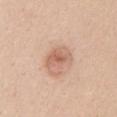biopsy status: total-body-photography surveillance lesion; no biopsy | body site: the mid back | automated lesion analysis: a footprint of about 7.5 mm² and an eccentricity of roughly 0.75 | illumination: white-light | acquisition: ~15 mm crop, total-body skin-cancer survey | subject: female, aged approximately 25 | lesion diameter: about 3.5 mm.